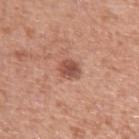biopsy status = catalogued during a skin exam; not biopsied
imaging modality = ~15 mm tile from a whole-body skin photo
patient = male, aged 48 to 52
lesion diameter = about 2.5 mm
location = the arm